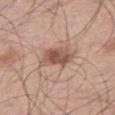The lesion is on the right thigh. A 15 mm close-up tile from a total-body photography series done for melanoma screening. A male subject about 70 years old.